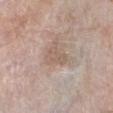Clinical impression:
Recorded during total-body skin imaging; not selected for excision or biopsy.
Acquisition and patient details:
The lesion is on the leg. A region of skin cropped from a whole-body photographic capture, roughly 15 mm wide. The patient is a female approximately 75 years of age. The lesion's longest dimension is about 3 mm. The tile uses white-light illumination.